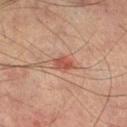follow-up = imaged on a skin check; not biopsied
body site = the left lower leg
image = ~15 mm tile from a whole-body skin photo
subject = male, in their mid- to late 70s